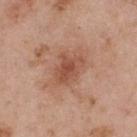Assessment:
No biopsy was performed on this lesion — it was imaged during a full skin examination and was not determined to be concerning.
Background:
Cropped from a total-body skin-imaging series; the visible field is about 15 mm. The patient is a male roughly 55 years of age. The lesion is located on the upper back.